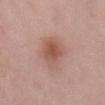| feature | finding |
|---|---|
| workup | imaged on a skin check; not biopsied |
| image | 15 mm crop, total-body photography |
| tile lighting | white-light |
| subject | female, aged around 65 |
| anatomic site | the lower back |